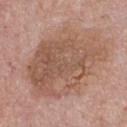The tile uses white-light illumination.
The patient is a male in their mid-70s.
The total-body-photography lesion software estimated a lesion area of about 55 mm², an eccentricity of roughly 0.7, and a symmetry-axis asymmetry near 0.2. It also reported a lesion color around L≈54 a*≈19 b*≈28 in CIELAB, about 9 CIELAB-L* units darker than the surrounding skin, and a lesion-to-skin contrast of about 6.5 (normalized; higher = more distinct). The analysis additionally found a nevus-likeness score of about 0/100 and lesion-presence confidence of about 100/100.
Measured at roughly 10 mm in maximum diameter.
This image is a 15 mm lesion crop taken from a total-body photograph.
Located on the chest.